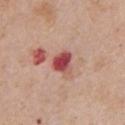{"biopsy_status": "not biopsied; imaged during a skin examination", "site": "chest", "patient": {"sex": "male", "age_approx": 60}, "lesion_size": {"long_diameter_mm_approx": 3.0}, "lighting": "white-light", "image": {"source": "total-body photography crop", "field_of_view_mm": 15}}